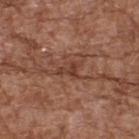No biopsy was performed on this lesion — it was imaged during a full skin examination and was not determined to be concerning. A 15 mm close-up extracted from a 3D total-body photography capture. The tile uses white-light illumination. A male subject, aged approximately 75. Longest diameter approximately 3 mm. Automated image analysis of the tile measured a nevus-likeness score of about 0/100. The lesion is on the upper back.